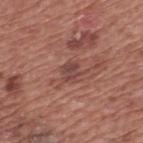{
  "biopsy_status": "not biopsied; imaged during a skin examination",
  "patient": {
    "sex": "male",
    "age_approx": 75
  },
  "site": "upper back",
  "image": {
    "source": "total-body photography crop",
    "field_of_view_mm": 15
  }
}